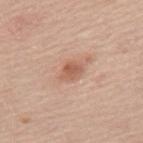• tile lighting · white-light
• size · ~2.5 mm (longest diameter)
• automated lesion analysis · an eccentricity of roughly 0.7 and two-axis asymmetry of about 0.35; roughly 10 lightness units darker than nearby skin and a normalized border contrast of about 7; a nevus-likeness score of about 70/100 and lesion-presence confidence of about 100/100
• image · ~15 mm crop, total-body skin-cancer survey
• subject · female, aged around 65
• body site · the mid back